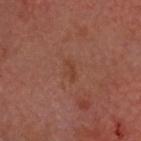No biopsy was performed on this lesion — it was imaged during a full skin examination and was not determined to be concerning.
Imaged with cross-polarized lighting.
The lesion is on the head or neck.
An algorithmic analysis of the crop reported a footprint of about 3 mm², a shape eccentricity near 0.85, and two-axis asymmetry of about 0.35. The analysis additionally found border irregularity of about 3.5 on a 0–10 scale, a color-variation rating of about 1.5/10, and peripheral color asymmetry of about 1. The analysis additionally found a nevus-likeness score of about 0/100 and lesion-presence confidence of about 100/100.
The lesion's longest dimension is about 2.5 mm.
Cropped from a total-body skin-imaging series; the visible field is about 15 mm.
A male patient, approximately 60 years of age.